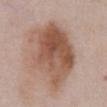• workup · catalogued during a skin exam; not biopsied
• subject · male, roughly 55 years of age
• TBP lesion metrics · an area of roughly 40 mm², an eccentricity of roughly 0.7, and a shape-asymmetry score of about 0.25 (0 = symmetric); an average lesion color of about L≈54 a*≈19 b*≈28 (CIELAB) and about 12 CIELAB-L* units darker than the surrounding skin
• lighting · white-light illumination
• body site · the chest
• size · ≈9 mm
• acquisition · ~15 mm crop, total-body skin-cancer survey